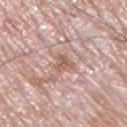* workup · no biopsy performed (imaged during a skin exam)
* patient · male, aged around 65
* size · ≈2.5 mm
* lighting · white-light
* anatomic site · the right upper arm
* imaging modality · ~15 mm crop, total-body skin-cancer survey